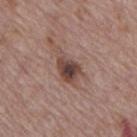No biopsy was performed on this lesion — it was imaged during a full skin examination and was not determined to be concerning. The recorded lesion diameter is about 5 mm. This image is a 15 mm lesion crop taken from a total-body photograph. A male subject, aged 68–72. The lesion is located on the back. Captured under white-light illumination.